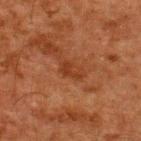No biopsy was performed on this lesion — it was imaged during a full skin examination and was not determined to be concerning. The subject is a male aged 58 to 62. The lesion is on the back. A 15 mm close-up tile from a total-body photography series done for melanoma screening. The lesion-visualizer software estimated an eccentricity of roughly 0.85 and a shape-asymmetry score of about 0.45 (0 = symmetric). The software also gave an average lesion color of about L≈29 a*≈23 b*≈29 (CIELAB). And it measured a classifier nevus-likeness of about 0/100 and a lesion-detection confidence of about 100/100. The tile uses cross-polarized illumination.